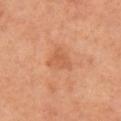Captured during whole-body skin photography for melanoma surveillance; the lesion was not biopsied.
A lesion tile, about 15 mm wide, cut from a 3D total-body photograph.
Approximately 3 mm at its widest.
From the left arm.
A female subject aged around 65.